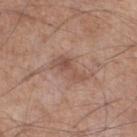The lesion was photographed on a routine skin check and not biopsied; there is no pathology result.
Approximately 4 mm at its widest.
Cropped from a whole-body photographic skin survey; the tile spans about 15 mm.
Imaged with white-light lighting.
A male patient, roughly 55 years of age.
From the left lower leg.